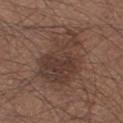The lesion was photographed on a routine skin check and not biopsied; there is no pathology result.
From the right thigh.
A male subject, aged 43 to 47.
A close-up tile cropped from a whole-body skin photograph, about 15 mm across.
Automated tile analysis of the lesion measured an average lesion color of about L≈37 a*≈17 b*≈22 (CIELAB) and a lesion-to-skin contrast of about 7 (normalized; higher = more distinct). The software also gave a border-irregularity rating of about 5.5/10.
Captured under white-light illumination.
About 8 mm across.